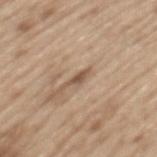biopsy_status: not biopsied; imaged during a skin examination
patient:
  sex: male
  age_approx: 70
image:
  source: total-body photography crop
  field_of_view_mm: 15
site: mid back